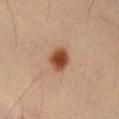The lesion was tiled from a total-body skin photograph and was not biopsied. About 3 mm across. Automated tile analysis of the lesion measured a footprint of about 6.5 mm² and a shape eccentricity near 0.6. It also reported a mean CIELAB color near L≈46 a*≈23 b*≈33 and a normalized lesion–skin contrast near 11. Captured under cross-polarized illumination. This image is a 15 mm lesion crop taken from a total-body photograph. A male patient aged 53 to 57. On the abdomen.